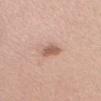Clinical impression: This lesion was catalogued during total-body skin photography and was not selected for biopsy. Acquisition and patient details: The lesion-visualizer software estimated an eccentricity of roughly 0.7 and two-axis asymmetry of about 0.25. The software also gave an average lesion color of about L≈58 a*≈22 b*≈27 (CIELAB) and a lesion–skin lightness drop of about 12. Longest diameter approximately 2.5 mm. The lesion is located on the head or neck. Imaged with white-light lighting. The subject is a male aged 23–27. A region of skin cropped from a whole-body photographic capture, roughly 15 mm wide.